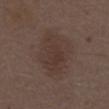{
  "biopsy_status": "not biopsied; imaged during a skin examination",
  "lesion_size": {
    "long_diameter_mm_approx": 6.5
  },
  "patient": {
    "sex": "male",
    "age_approx": 70
  },
  "image": {
    "source": "total-body photography crop",
    "field_of_view_mm": 15
  },
  "site": "abdomen"
}